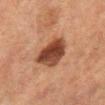Recorded during total-body skin imaging; not selected for excision or biopsy. Imaged with cross-polarized lighting. A male patient approximately 70 years of age. Automated tile analysis of the lesion measured a lesion area of about 14 mm², a shape eccentricity near 0.4, and two-axis asymmetry of about 0.25. And it measured a color-variation rating of about 5.5/10 and peripheral color asymmetry of about 1.5. A 15 mm crop from a total-body photograph taken for skin-cancer surveillance. From the left lower leg. The lesion's longest dimension is about 4.5 mm.